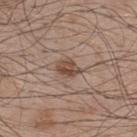Imaged during a routine full-body skin examination; the lesion was not biopsied and no histopathology is available.
The recorded lesion diameter is about 2.5 mm.
From the upper back.
The tile uses white-light illumination.
Cropped from a total-body skin-imaging series; the visible field is about 15 mm.
A male subject, aged approximately 50.